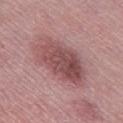Recorded during total-body skin imaging; not selected for excision or biopsy. The lesion is on the left thigh. Cropped from a total-body skin-imaging series; the visible field is about 15 mm. Approximately 7.5 mm at its widest. The lesion-visualizer software estimated a shape-asymmetry score of about 0.15 (0 = symmetric). It also reported a border-irregularity index near 2/10, internal color variation of about 5.5 on a 0–10 scale, and peripheral color asymmetry of about 2. The tile uses white-light illumination. A female patient, roughly 45 years of age.